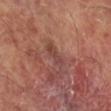biopsy_status: not biopsied; imaged during a skin examination
site: leg
patient:
  sex: male
  age_approx: 65
image:
  source: total-body photography crop
  field_of_view_mm: 15
lighting: cross-polarized
lesion_size:
  long_diameter_mm_approx: 3.5